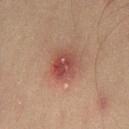Q: Is there a histopathology result?
A: no biopsy performed (imaged during a skin exam)